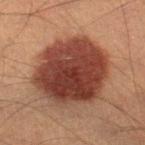Findings:
* location: the right lower leg
* patient: male, aged approximately 40
* image: ~15 mm crop, total-body skin-cancer survey
* tile lighting: cross-polarized
* size: ~8 mm (longest diameter)
* image-analysis metrics: an automated nevus-likeness rating near 100 out of 100 and lesion-presence confidence of about 100/100